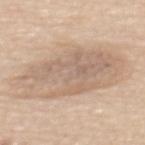workup = catalogued during a skin exam; not biopsied
anatomic site = the mid back
lesion diameter = ≈11 mm
patient = female, aged 63–67
automated lesion analysis = an area of roughly 49 mm², a shape eccentricity near 0.85, and a symmetry-axis asymmetry near 0.2; a detector confidence of about 85 out of 100 that the crop contains a lesion
acquisition = 15 mm crop, total-body photography